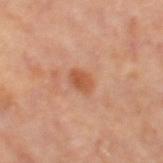Assessment: Part of a total-body skin-imaging series; this lesion was reviewed on a skin check and was not flagged for biopsy. Clinical summary: The recorded lesion diameter is about 2.5 mm. Cropped from a whole-body photographic skin survey; the tile spans about 15 mm. The total-body-photography lesion software estimated an average lesion color of about L≈52 a*≈25 b*≈35 (CIELAB) and about 9 CIELAB-L* units darker than the surrounding skin. A female subject, aged approximately 50. Captured under cross-polarized illumination. The lesion is located on the right thigh.